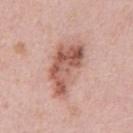Imaged during a routine full-body skin examination; the lesion was not biopsied and no histopathology is available. The subject is a male aged 38 to 42. A close-up tile cropped from a whole-body skin photograph, about 15 mm across. Measured at roughly 7 mm in maximum diameter. Imaged with white-light lighting. Located on the abdomen.